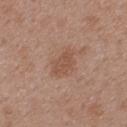The lesion was tiled from a total-body skin photograph and was not biopsied. Located on the back. A female subject aged approximately 40. The lesion's longest dimension is about 4 mm. Captured under white-light illumination. A 15 mm crop from a total-body photograph taken for skin-cancer surveillance. The lesion-visualizer software estimated an eccentricity of roughly 0.8 and two-axis asymmetry of about 0.3. The software also gave an average lesion color of about L≈51 a*≈20 b*≈29 (CIELAB), about 7 CIELAB-L* units darker than the surrounding skin, and a normalized border contrast of about 6. It also reported border irregularity of about 3.5 on a 0–10 scale, internal color variation of about 1.5 on a 0–10 scale, and radial color variation of about 0.5. It also reported an automated nevus-likeness rating near 15 out of 100 and a detector confidence of about 100 out of 100 that the crop contains a lesion.